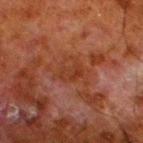Assessment:
Imaged during a routine full-body skin examination; the lesion was not biopsied and no histopathology is available.
Image and clinical context:
A close-up tile cropped from a whole-body skin photograph, about 15 mm across. The lesion is on the left lower leg. The recorded lesion diameter is about 4 mm. A male patient, aged approximately 80.